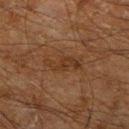A roughly 15 mm field-of-view crop from a total-body skin photograph. A male subject, aged 58 to 62. On the right lower leg. Longest diameter approximately 4.5 mm. Automated image analysis of the tile measured a border-irregularity rating of about 6/10, a color-variation rating of about 1/10, and a peripheral color-asymmetry measure near 0.5. The software also gave a nevus-likeness score of about 10/100.